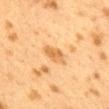notes = total-body-photography surveillance lesion; no biopsy
image = ~15 mm tile from a whole-body skin photo
patient = female, aged 38 to 42
anatomic site = the back